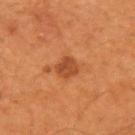<case>
  <biopsy_status>not biopsied; imaged during a skin examination</biopsy_status>
  <image>
    <source>total-body photography crop</source>
    <field_of_view_mm>15</field_of_view_mm>
  </image>
  <lighting>cross-polarized</lighting>
  <lesion_size>
    <long_diameter_mm_approx>2.5</long_diameter_mm_approx>
  </lesion_size>
  <site>left upper arm</site>
  <automated_metrics>
    <area_mm2_approx>5.0</area_mm2_approx>
    <eccentricity>0.45</eccentricity>
    <shape_asymmetry>0.25</shape_asymmetry>
    <cielab_L>44</cielab_L>
    <cielab_a>27</cielab_a>
    <cielab_b>37</cielab_b>
    <vs_skin_darker_L>9.0</vs_skin_darker_L>
    <vs_skin_contrast_norm>7.0</vs_skin_contrast_norm>
  </automated_metrics>
  <patient>
    <sex>male</sex>
    <age_approx>55</age_approx>
  </patient>
</case>